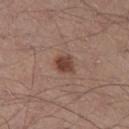From the left thigh.
This image is a 15 mm lesion crop taken from a total-body photograph.
A male subject about 55 years old.